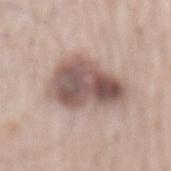location = the mid back | subject = male, approximately 65 years of age | lesion diameter = ≈7 mm | imaging modality = total-body-photography crop, ~15 mm field of view.